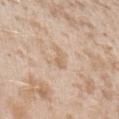notes: imaged on a skin check; not biopsied | patient: female, in their mid-20s | image source: ~15 mm crop, total-body skin-cancer survey | illumination: white-light illumination | automated lesion analysis: roughly 7 lightness units darker than nearby skin and a normalized border contrast of about 5; a nevus-likeness score of about 0/100 and a detector confidence of about 100 out of 100 that the crop contains a lesion | body site: the left upper arm.